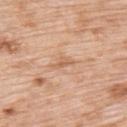Impression:
No biopsy was performed on this lesion — it was imaged during a full skin examination and was not determined to be concerning.
Image and clinical context:
Cropped from a whole-body photographic skin survey; the tile spans about 15 mm. On the upper back. The patient is a female aged around 70. The lesion's longest dimension is about 3 mm.